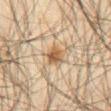Q: Was this lesion biopsied?
A: imaged on a skin check; not biopsied
Q: What is the lesion's diameter?
A: ~2.5 mm (longest diameter)
Q: Who is the patient?
A: male, in their mid-60s
Q: Lesion location?
A: the abdomen
Q: How was the tile lit?
A: cross-polarized illumination
Q: What did automated image analysis measure?
A: an area of roughly 5.5 mm², an eccentricity of roughly 0.4, and two-axis asymmetry of about 0.25; an average lesion color of about L≈48 a*≈14 b*≈30 (CIELAB), about 9 CIELAB-L* units darker than the surrounding skin, and a normalized border contrast of about 8; a border-irregularity index near 2/10, a within-lesion color-variation index near 4/10, and a peripheral color-asymmetry measure near 1.5
Q: What kind of image is this?
A: ~15 mm tile from a whole-body skin photo